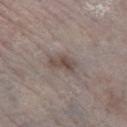The lesion was photographed on a routine skin check and not biopsied; there is no pathology result.
On the right lower leg.
A male subject, aged around 75.
A roughly 15 mm field-of-view crop from a total-body skin photograph.
About 3.5 mm across.